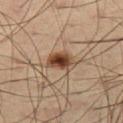Assessment: No biopsy was performed on this lesion — it was imaged during a full skin examination and was not determined to be concerning. Image and clinical context: The patient is a male in their mid-60s. On the left thigh. A close-up tile cropped from a whole-body skin photograph, about 15 mm across.